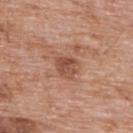follow-up: total-body-photography surveillance lesion; no biopsy
patient: male, approximately 60 years of age
location: the upper back
size: ~2.5 mm (longest diameter)
TBP lesion metrics: an eccentricity of roughly 0.35; a nevus-likeness score of about 25/100 and lesion-presence confidence of about 100/100
lighting: white-light
image: ~15 mm tile from a whole-body skin photo